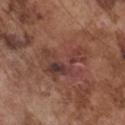{
  "site": "front of the torso",
  "image": {
    "source": "total-body photography crop",
    "field_of_view_mm": 15
  },
  "lesion_size": {
    "long_diameter_mm_approx": 5.5
  },
  "patient": {
    "sex": "male",
    "age_approx": 75
  },
  "lighting": "white-light"
}